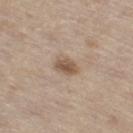Recorded during total-body skin imaging; not selected for excision or biopsy. The lesion is located on the right thigh. Cropped from a whole-body photographic skin survey; the tile spans about 15 mm. The recorded lesion diameter is about 3 mm. The tile uses white-light illumination. A male subject, aged 68 to 72.